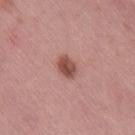Imaged during a routine full-body skin examination; the lesion was not biopsied and no histopathology is available.
Automated image analysis of the tile measured a lesion color around L≈50 a*≈25 b*≈25 in CIELAB, a lesion–skin lightness drop of about 13, and a lesion-to-skin contrast of about 9 (normalized; higher = more distinct). And it measured a border-irregularity index near 2/10 and a color-variation rating of about 4.5/10. The analysis additionally found an automated nevus-likeness rating near 95 out of 100 and lesion-presence confidence of about 100/100.
From the right thigh.
The tile uses white-light illumination.
A 15 mm close-up extracted from a 3D total-body photography capture.
Measured at roughly 3 mm in maximum diameter.
The subject is a female approximately 50 years of age.